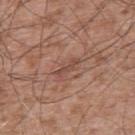Part of a total-body skin-imaging series; this lesion was reviewed on a skin check and was not flagged for biopsy.
From the upper back.
A 15 mm close-up extracted from a 3D total-body photography capture.
A male subject aged around 55.